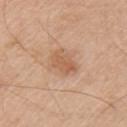Impression:
This lesion was catalogued during total-body skin photography and was not selected for biopsy.
Clinical summary:
The lesion is on the left upper arm. Captured under white-light illumination. An algorithmic analysis of the crop reported a lesion area of about 5.5 mm². The software also gave a mean CIELAB color near L≈58 a*≈21 b*≈33, about 9 CIELAB-L* units darker than the surrounding skin, and a normalized border contrast of about 6. And it measured a border-irregularity index near 3/10, a color-variation rating of about 2/10, and radial color variation of about 1. The analysis additionally found a classifier nevus-likeness of about 15/100 and lesion-presence confidence of about 100/100. A male patient approximately 70 years of age. Measured at roughly 3.5 mm in maximum diameter. Cropped from a total-body skin-imaging series; the visible field is about 15 mm.